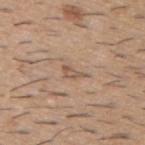Notes:
– workup · imaged on a skin check; not biopsied
– automated lesion analysis · an area of roughly 3 mm², a shape eccentricity near 0.9, and two-axis asymmetry of about 0.5; a lesion color around L≈55 a*≈17 b*≈28 in CIELAB, a lesion–skin lightness drop of about 9, and a normalized lesion–skin contrast near 6; a border-irregularity index near 5/10 and internal color variation of about 1 on a 0–10 scale
– anatomic site · the upper back
– image source · ~15 mm crop, total-body skin-cancer survey
– subject · male, aged 58 to 62
– lesion diameter · about 3 mm
– illumination · white-light illumination